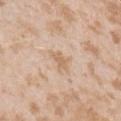Clinical impression: Recorded during total-body skin imaging; not selected for excision or biopsy. Clinical summary: The lesion is on the arm. A close-up tile cropped from a whole-body skin photograph, about 15 mm across. The subject is a female about 25 years old. Captured under white-light illumination.